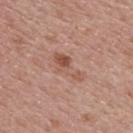workup: catalogued during a skin exam; not biopsied | acquisition: ~15 mm tile from a whole-body skin photo | patient: female, about 65 years old | tile lighting: white-light | location: the back | lesion size: about 4.5 mm | image-analysis metrics: an average lesion color of about L≈53 a*≈22 b*≈29 (CIELAB), roughly 8 lightness units darker than nearby skin, and a lesion-to-skin contrast of about 6.5 (normalized; higher = more distinct); internal color variation of about 4.5 on a 0–10 scale and peripheral color asymmetry of about 1.5.